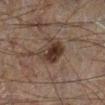Part of a total-body skin-imaging series; this lesion was reviewed on a skin check and was not flagged for biopsy. Automated image analysis of the tile measured roughly 9 lightness units darker than nearby skin and a normalized border contrast of about 10. It also reported a border-irregularity rating of about 3.5/10, a within-lesion color-variation index near 4/10, and a peripheral color-asymmetry measure near 1.5. The analysis additionally found an automated nevus-likeness rating near 90 out of 100 and lesion-presence confidence of about 100/100. The lesion is on the left lower leg. Measured at roughly 3.5 mm in maximum diameter. A 15 mm crop from a total-body photograph taken for skin-cancer surveillance. The subject is a male in their 60s.